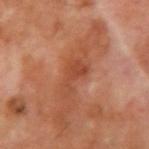Clinical impression:
This lesion was catalogued during total-body skin photography and was not selected for biopsy.
Acquisition and patient details:
A 15 mm crop from a total-body photograph taken for skin-cancer surveillance. The subject is a male aged 68 to 72. Automated tile analysis of the lesion measured an area of roughly 5.5 mm², an outline eccentricity of about 0.9 (0 = round, 1 = elongated), and two-axis asymmetry of about 0.6. And it measured a mean CIELAB color near L≈46 a*≈28 b*≈34, roughly 8 lightness units darker than nearby skin, and a lesion-to-skin contrast of about 6 (normalized; higher = more distinct). And it measured a classifier nevus-likeness of about 0/100. About 4 mm across. Located on the right upper arm.